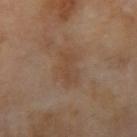The lesion was tiled from a total-body skin photograph and was not biopsied. A lesion tile, about 15 mm wide, cut from a 3D total-body photograph. Automated tile analysis of the lesion measured a border-irregularity rating of about 4/10, a color-variation rating of about 2.5/10, and peripheral color asymmetry of about 1. About 4 mm across. From the right upper arm. A male patient aged approximately 70. The tile uses cross-polarized illumination.